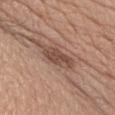The lesion was photographed on a routine skin check and not biopsied; there is no pathology result.
A male patient, aged 63–67.
On the chest.
This is a white-light tile.
About 4 mm across.
Cropped from a whole-body photographic skin survey; the tile spans about 15 mm.